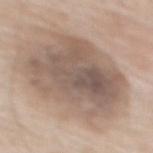Notes:
– notes: no biopsy performed (imaged during a skin exam)
– TBP lesion metrics: about 12 CIELAB-L* units darker than the surrounding skin and a normalized border contrast of about 8; border irregularity of about 2.5 on a 0–10 scale and a color-variation rating of about 6.5/10
– lesion size: ~9 mm (longest diameter)
– lighting: white-light
– image source: total-body-photography crop, ~15 mm field of view
– subject: male, aged 63 to 67
– body site: the mid back